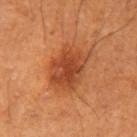Imaged with cross-polarized lighting.
A lesion tile, about 15 mm wide, cut from a 3D total-body photograph.
Located on the left upper arm.
The recorded lesion diameter is about 5.5 mm.
The subject is a male approximately 60 years of age.